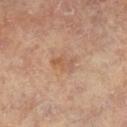tile lighting — cross-polarized illumination
site — the leg
subject — female, in their mid-70s
image — 15 mm crop, total-body photography
automated metrics — a border-irregularity index near 3.5/10, a color-variation rating of about 3.5/10, and a peripheral color-asymmetry measure near 1; an automated nevus-likeness rating near 0 out of 100
diameter — ≈3 mm